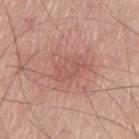Q: What is the lesion's diameter?
A: about 4 mm
Q: Automated lesion metrics?
A: a footprint of about 7 mm², an outline eccentricity of about 0.85 (0 = round, 1 = elongated), and a shape-asymmetry score of about 0.35 (0 = symmetric); a lesion color around L≈55 a*≈25 b*≈25 in CIELAB and a normalized lesion–skin contrast near 4.5; a nevus-likeness score of about 5/100 and lesion-presence confidence of about 100/100
Q: What kind of image is this?
A: ~15 mm tile from a whole-body skin photo
Q: Lesion location?
A: the left thigh
Q: Illumination type?
A: white-light illumination
Q: Who is the patient?
A: male, aged 68–72